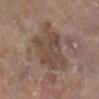<lesion>
  <biopsy_status>not biopsied; imaged during a skin examination</biopsy_status>
  <patient>
    <sex>female</sex>
    <age_approx>85+</age_approx>
  </patient>
  <site>right lower leg</site>
  <image>
    <source>total-body photography crop</source>
    <field_of_view_mm>15</field_of_view_mm>
  </image>
</lesion>